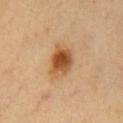workup = catalogued during a skin exam; not biopsied | lighting = cross-polarized | subject = male, aged around 50 | image = total-body-photography crop, ~15 mm field of view | lesion size = about 4 mm | automated lesion analysis = a lesion color around L≈53 a*≈22 b*≈39 in CIELAB, a lesion–skin lightness drop of about 13, and a normalized border contrast of about 9.5; a peripheral color-asymmetry measure near 2; a nevus-likeness score of about 100/100 and lesion-presence confidence of about 100/100 | site = the chest.